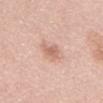{"biopsy_status": "not biopsied; imaged during a skin examination", "site": "abdomen", "image": {"source": "total-body photography crop", "field_of_view_mm": 15}, "lesion_size": {"long_diameter_mm_approx": 3.0}, "patient": {"sex": "male", "age_approx": 55}, "lighting": "white-light", "automated_metrics": {"cielab_L": 65, "cielab_a": 22, "cielab_b": 28, "vs_skin_contrast_norm": 6.0}}